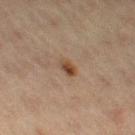{"biopsy_status": "not biopsied; imaged during a skin examination", "patient": {"sex": "female", "age_approx": 65}, "site": "left thigh", "image": {"source": "total-body photography crop", "field_of_view_mm": 15}, "automated_metrics": {"cielab_L": 39, "cielab_a": 16, "cielab_b": 28, "vs_skin_contrast_norm": 9.0, "color_variation_0_10": 5.5, "peripheral_color_asymmetry": 2.0, "nevus_likeness_0_100": 95, "lesion_detection_confidence_0_100": 100}}